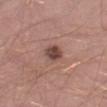biopsy status: total-body-photography surveillance lesion; no biopsy
image: total-body-photography crop, ~15 mm field of view
subject: male, approximately 40 years of age
location: the left thigh
lighting: white-light
TBP lesion metrics: an area of roughly 5 mm² and an eccentricity of roughly 0.55
size: ≈2.5 mm